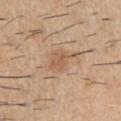The lesion was tiled from a total-body skin photograph and was not biopsied. Longest diameter approximately 4.5 mm. Captured under white-light illumination. A 15 mm close-up tile from a total-body photography series done for melanoma screening. The subject is a male aged 58–62. From the left upper arm.